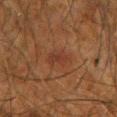Case summary:
- workup: catalogued during a skin exam; not biopsied
- imaging modality: ~15 mm crop, total-body skin-cancer survey
- lesion diameter: about 3.5 mm
- subject: male, about 65 years old
- illumination: cross-polarized
- anatomic site: the right forearm